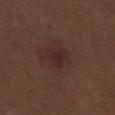Recorded during total-body skin imaging; not selected for excision or biopsy. A close-up tile cropped from a whole-body skin photograph, about 15 mm across. About 3 mm across. On the right lower leg. Imaged with white-light lighting. The subject is a male aged around 70.